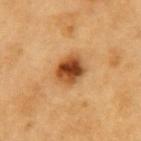Impression: Recorded during total-body skin imaging; not selected for excision or biopsy. Context: Approximately 3.5 mm at its widest. The patient is a male approximately 60 years of age. This is a cross-polarized tile. Cropped from a total-body skin-imaging series; the visible field is about 15 mm. Located on the left upper arm.